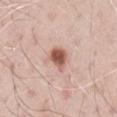Q: What kind of image is this?
A: 15 mm crop, total-body photography
Q: Patient demographics?
A: male, aged approximately 70
Q: Illumination type?
A: white-light illumination
Q: How large is the lesion?
A: ≈2.5 mm
Q: Where on the body is the lesion?
A: the right upper arm